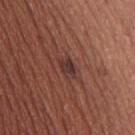The lesion-visualizer software estimated a footprint of about 4 mm², an outline eccentricity of about 0.75 (0 = round, 1 = elongated), and a shape-asymmetry score of about 0.3 (0 = symmetric). It also reported a lesion-to-skin contrast of about 8.5 (normalized; higher = more distinct). And it measured an automated nevus-likeness rating near 0 out of 100 and lesion-presence confidence of about 100/100. A region of skin cropped from a whole-body photographic capture, roughly 15 mm wide. On the upper back. A male subject, in their mid- to late 40s. Measured at roughly 2.5 mm in maximum diameter.